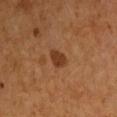No biopsy was performed on this lesion — it was imaged during a full skin examination and was not determined to be concerning. Measured at roughly 2.5 mm in maximum diameter. This is a cross-polarized tile. A male patient aged approximately 55. From the arm. An algorithmic analysis of the crop reported an area of roughly 4 mm², an eccentricity of roughly 0.75, and two-axis asymmetry of about 0.2. The software also gave an average lesion color of about L≈34 a*≈22 b*≈31 (CIELAB) and a normalized lesion–skin contrast near 9. The software also gave a border-irregularity rating of about 2/10 and peripheral color asymmetry of about 0.5. A roughly 15 mm field-of-view crop from a total-body skin photograph.